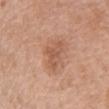Assessment: The lesion was photographed on a routine skin check and not biopsied; there is no pathology result. Background: A male patient about 65 years old. Cropped from a whole-body photographic skin survey; the tile spans about 15 mm. Located on the chest.